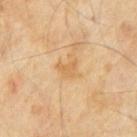Clinical impression: Imaged during a routine full-body skin examination; the lesion was not biopsied and no histopathology is available. Acquisition and patient details: A male patient, aged 58–62. About 2.5 mm across. Captured under cross-polarized illumination. A 15 mm close-up extracted from a 3D total-body photography capture. On the abdomen.